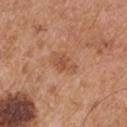Clinical impression:
No biopsy was performed on this lesion — it was imaged during a full skin examination and was not determined to be concerning.